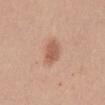Case summary:
* follow-up — no biopsy performed (imaged during a skin exam)
* patient — female, aged approximately 35
* image — ~15 mm crop, total-body skin-cancer survey
* automated lesion analysis — an area of roughly 5 mm², an outline eccentricity of about 0.8 (0 = round, 1 = elongated), and a symmetry-axis asymmetry near 0.2; a lesion color around L≈57 a*≈23 b*≈30 in CIELAB, roughly 11 lightness units darker than nearby skin, and a normalized border contrast of about 7; a border-irregularity index near 2/10, a color-variation rating of about 2/10, and a peripheral color-asymmetry measure near 0.5; a classifier nevus-likeness of about 95/100 and a detector confidence of about 100 out of 100 that the crop contains a lesion
* illumination — white-light
* site — the abdomen
* size — about 3 mm